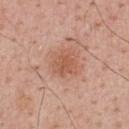Q: Is there a histopathology result?
A: total-body-photography surveillance lesion; no biopsy
Q: What kind of image is this?
A: ~15 mm crop, total-body skin-cancer survey
Q: How large is the lesion?
A: about 3 mm
Q: How was the tile lit?
A: white-light
Q: Where on the body is the lesion?
A: the upper back
Q: Patient demographics?
A: male, aged approximately 65